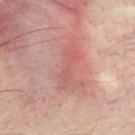Q: Is there a histopathology result?
A: catalogued during a skin exam; not biopsied
Q: How was the tile lit?
A: cross-polarized
Q: What kind of image is this?
A: ~15 mm crop, total-body skin-cancer survey
Q: What are the patient's age and sex?
A: male, approximately 35 years of age
Q: What is the anatomic site?
A: the chest
Q: What is the lesion's diameter?
A: ~5 mm (longest diameter)